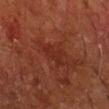  automated_metrics:
    border_irregularity_0_10: 5.5
    peripheral_color_asymmetry: 0.5
    nevus_likeness_0_100: 0
    lesion_detection_confidence_0_100: 100
  patient:
    sex: male
    age_approx: 60
  lesion_size:
    long_diameter_mm_approx: 3.5
  lighting: cross-polarized
  image:
    source: total-body photography crop
    field_of_view_mm: 15
  site: right forearm